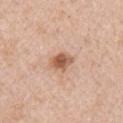– follow-up · no biopsy performed (imaged during a skin exam)
– lesion size · ~3 mm (longest diameter)
– subject · female, approximately 70 years of age
– image source · ~15 mm crop, total-body skin-cancer survey
– illumination · white-light
– body site · the right upper arm